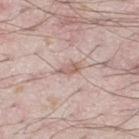Approximately 3 mm at its widest. A region of skin cropped from a whole-body photographic capture, roughly 15 mm wide. Captured under white-light illumination. The lesion is on the left thigh. A male patient, in their mid- to late 20s.